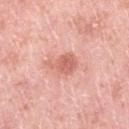biopsy status: no biopsy performed (imaged during a skin exam) | subject: female, aged around 40 | anatomic site: the leg | lesion diameter: about 4 mm | image: ~15 mm crop, total-body skin-cancer survey.